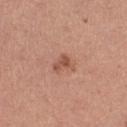biopsy_status: not biopsied; imaged during a skin examination
site: right thigh
lighting: white-light
patient:
  sex: female
  age_approx: 30
automated_metrics:
  area_mm2_approx: 3.5
  eccentricity: 0.65
  shape_asymmetry: 0.45
  border_irregularity_0_10: 4.5
  color_variation_0_10: 2.5
  peripheral_color_asymmetry: 1.0
lesion_size:
  long_diameter_mm_approx: 2.5
image:
  source: total-body photography crop
  field_of_view_mm: 15